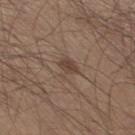Q: How large is the lesion?
A: ≈3.5 mm
Q: What is the anatomic site?
A: the leg
Q: How was this image acquired?
A: 15 mm crop, total-body photography
Q: How was the tile lit?
A: white-light illumination
Q: Who is the patient?
A: male, roughly 30 years of age
Q: What did automated image analysis measure?
A: an area of roughly 5 mm², a shape eccentricity near 0.7, and two-axis asymmetry of about 0.35; roughly 7 lightness units darker than nearby skin and a lesion-to-skin contrast of about 6 (normalized; higher = more distinct); a within-lesion color-variation index near 2/10 and peripheral color asymmetry of about 0.5